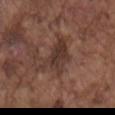Assessment:
Captured during whole-body skin photography for melanoma surveillance; the lesion was not biopsied.
Acquisition and patient details:
Located on the mid back. A male subject aged 73 to 77. A 15 mm close-up tile from a total-body photography series done for melanoma screening. The lesion-visualizer software estimated a footprint of about 9.5 mm², an outline eccentricity of about 0.8 (0 = round, 1 = elongated), and two-axis asymmetry of about 0.4. The analysis additionally found a lesion color around L≈34 a*≈18 b*≈22 in CIELAB and about 9 CIELAB-L* units darker than the surrounding skin. The software also gave a within-lesion color-variation index near 3/10 and peripheral color asymmetry of about 1. And it measured lesion-presence confidence of about 80/100. Approximately 5 mm at its widest.